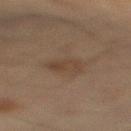notes: imaged on a skin check; not biopsied
anatomic site: the right lower leg
automated metrics: an outline eccentricity of about 0.8 (0 = round, 1 = elongated) and a symmetry-axis asymmetry near 0.25; an average lesion color of about L≈36 a*≈13 b*≈24 (CIELAB) and a normalized border contrast of about 5.5; lesion-presence confidence of about 100/100
subject: female, aged 53 to 57
illumination: cross-polarized illumination
imaging modality: 15 mm crop, total-body photography
diameter: about 3.5 mm